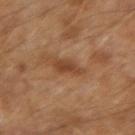Q: Automated lesion metrics?
A: a lesion color around L≈42 a*≈21 b*≈33 in CIELAB; a within-lesion color-variation index near 2/10 and radial color variation of about 0.5
Q: Patient demographics?
A: aged approximately 55
Q: What is the anatomic site?
A: the right forearm
Q: What lighting was used for the tile?
A: cross-polarized
Q: How was this image acquired?
A: 15 mm crop, total-body photography